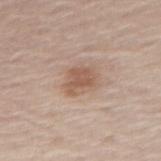<lesion>
<biopsy_status>not biopsied; imaged during a skin examination</biopsy_status>
<patient>
  <sex>male</sex>
  <age_approx>80</age_approx>
</patient>
<site>right thigh</site>
<automated_metrics>
  <border_irregularity_0_10>3.0</border_irregularity_0_10>
  <color_variation_0_10>2.0</color_variation_0_10>
  <peripheral_color_asymmetry>0.5</peripheral_color_asymmetry>
  <nevus_likeness_0_100>55</nevus_likeness_0_100>
</automated_metrics>
<lesion_size>
  <long_diameter_mm_approx>3.5</long_diameter_mm_approx>
</lesion_size>
<lighting>white-light</lighting>
<image>
  <source>total-body photography crop</source>
  <field_of_view_mm>15</field_of_view_mm>
</image>
</lesion>